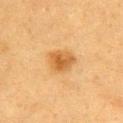The lesion was tiled from a total-body skin photograph and was not biopsied. The lesion's longest dimension is about 3.5 mm. The lesion is on the upper back. The tile uses cross-polarized illumination. The total-body-photography lesion software estimated a footprint of about 7.5 mm², an outline eccentricity of about 0.65 (0 = round, 1 = elongated), and a symmetry-axis asymmetry near 0.2. The software also gave a classifier nevus-likeness of about 90/100 and lesion-presence confidence of about 100/100. A 15 mm crop from a total-body photograph taken for skin-cancer surveillance. The patient is a female aged around 40.